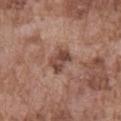Impression: Recorded during total-body skin imaging; not selected for excision or biopsy. Context: The patient is a male approximately 75 years of age. Captured under white-light illumination. From the abdomen. A close-up tile cropped from a whole-body skin photograph, about 15 mm across.